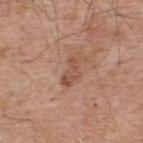This lesion was catalogued during total-body skin photography and was not selected for biopsy. This is a white-light tile. Located on the upper back. A male subject roughly 55 years of age. About 3.5 mm across. A 15 mm close-up extracted from a 3D total-body photography capture.